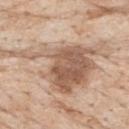Imaged during a routine full-body skin examination; the lesion was not biopsied and no histopathology is available.
A 15 mm close-up tile from a total-body photography series done for melanoma screening.
The subject is a male roughly 85 years of age or older.
The lesion's longest dimension is about 12.5 mm.
The lesion is on the upper back.
The tile uses white-light illumination.